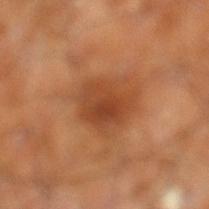Impression:
Recorded during total-body skin imaging; not selected for excision or biopsy.